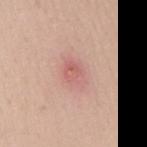Q: Is there a histopathology result?
A: total-body-photography surveillance lesion; no biopsy
Q: How was the tile lit?
A: white-light illumination
Q: How was this image acquired?
A: 15 mm crop, total-body photography
Q: What are the patient's age and sex?
A: female, in their 30s
Q: What is the lesion's diameter?
A: about 3.5 mm
Q: What is the anatomic site?
A: the mid back